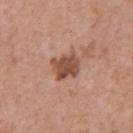{
  "biopsy_status": "not biopsied; imaged during a skin examination",
  "lighting": "white-light",
  "site": "chest",
  "image": {
    "source": "total-body photography crop",
    "field_of_view_mm": 15
  },
  "patient": {
    "sex": "male",
    "age_approx": 70
  }
}